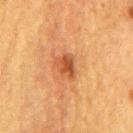  biopsy_status: not biopsied; imaged during a skin examination
  automated_metrics:
    area_mm2_approx: 5.5
    eccentricity: 0.65
    shape_asymmetry: 0.3
    border_irregularity_0_10: 3.0
    color_variation_0_10: 4.0
    peripheral_color_asymmetry: 1.5
  patient:
    sex: female
    age_approx: 55
  site: chest
  lesion_size:
    long_diameter_mm_approx: 3.0
  lighting: cross-polarized
  image:
    source: total-body photography crop
    field_of_view_mm: 15